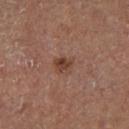follow-up — catalogued during a skin exam; not biopsied
tile lighting — cross-polarized illumination
TBP lesion metrics — an outline eccentricity of about 0.5 (0 = round, 1 = elongated) and two-axis asymmetry of about 0.3; a color-variation rating of about 4/10 and peripheral color asymmetry of about 1.5; an automated nevus-likeness rating near 85 out of 100
patient — male, approximately 65 years of age
size — ≈2.5 mm
acquisition — ~15 mm tile from a whole-body skin photo
body site — the right lower leg